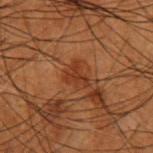Impression: The lesion was tiled from a total-body skin photograph and was not biopsied.